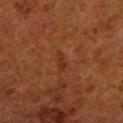Q: Was this lesion biopsied?
A: imaged on a skin check; not biopsied
Q: What are the patient's age and sex?
A: male, aged around 80
Q: What kind of image is this?
A: total-body-photography crop, ~15 mm field of view
Q: What lighting was used for the tile?
A: cross-polarized illumination
Q: Automated lesion metrics?
A: an area of roughly 2.5 mm², an outline eccentricity of about 0.95 (0 = round, 1 = elongated), and two-axis asymmetry of about 0.4
Q: What is the lesion's diameter?
A: ~3 mm (longest diameter)
Q: Where on the body is the lesion?
A: the right lower leg